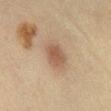The lesion was tiled from a total-body skin photograph and was not biopsied.
A lesion tile, about 15 mm wide, cut from a 3D total-body photograph.
On the right lower leg.
Captured under cross-polarized illumination.
A female subject, roughly 65 years of age.